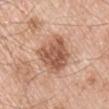follow-up: imaged on a skin check; not biopsied | size: ≈5 mm | body site: the left upper arm | patient: male, aged approximately 55 | image: ~15 mm crop, total-body skin-cancer survey.